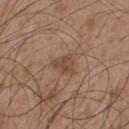Q: Was a biopsy performed?
A: imaged on a skin check; not biopsied
Q: Where on the body is the lesion?
A: the upper back
Q: What kind of image is this?
A: ~15 mm crop, total-body skin-cancer survey
Q: What are the patient's age and sex?
A: male, approximately 50 years of age
Q: What lighting was used for the tile?
A: white-light illumination
Q: How large is the lesion?
A: ~3 mm (longest diameter)